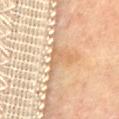Impression:
Part of a total-body skin-imaging series; this lesion was reviewed on a skin check and was not flagged for biopsy.
Acquisition and patient details:
Cropped from a total-body skin-imaging series; the visible field is about 15 mm. The recorded lesion diameter is about 4 mm. Imaged with cross-polarized lighting. A female patient, in their 60s. The lesion is on the mid back.